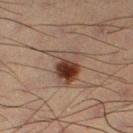workup = catalogued during a skin exam; not biopsied
body site = the left thigh
lesion size = ~4.5 mm (longest diameter)
tile lighting = cross-polarized
image = ~15 mm tile from a whole-body skin photo
subject = male, in their mid- to late 50s
TBP lesion metrics = border irregularity of about 4 on a 0–10 scale and a color-variation rating of about 10/10